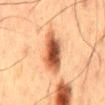Notes:
• notes — total-body-photography surveillance lesion; no biopsy
• patient — male, in their 60s
• tile lighting — cross-polarized
• anatomic site — the back
• image-analysis metrics — a classifier nevus-likeness of about 100/100 and a detector confidence of about 100 out of 100 that the crop contains a lesion
• diameter — ~5.5 mm (longest diameter)
• acquisition — ~15 mm tile from a whole-body skin photo